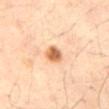Assessment: The lesion was tiled from a total-body skin photograph and was not biopsied. Acquisition and patient details: A 15 mm crop from a total-body photograph taken for skin-cancer surveillance. Captured under cross-polarized illumination. Automated image analysis of the tile measured border irregularity of about 1.5 on a 0–10 scale and peripheral color asymmetry of about 1. The analysis additionally found a nevus-likeness score of about 100/100 and a lesion-detection confidence of about 100/100. On the abdomen. A male subject about 65 years old.